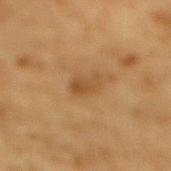notes = imaged on a skin check; not biopsied
subject = male, in their mid- to late 80s or older
lesion size = ≈3 mm
image = 15 mm crop, total-body photography
location = the mid back